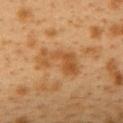Clinical impression:
The lesion was photographed on a routine skin check and not biopsied; there is no pathology result.
Context:
A lesion tile, about 15 mm wide, cut from a 3D total-body photograph. Imaged with cross-polarized lighting. The lesion is located on the upper back. The subject is a female aged 38–42. Approximately 5.5 mm at its widest.